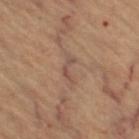Clinical impression:
Recorded during total-body skin imaging; not selected for excision or biopsy.
Context:
A 15 mm close-up tile from a total-body photography series done for melanoma screening. Captured under cross-polarized illumination. The subject is a female aged 58 to 62. The lesion is located on the left thigh.